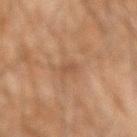Part of a total-body skin-imaging series; this lesion was reviewed on a skin check and was not flagged for biopsy. The lesion's longest dimension is about 2.5 mm. An algorithmic analysis of the crop reported an eccentricity of roughly 0.9 and a symmetry-axis asymmetry near 0.35. It also reported a lesion color around L≈41 a*≈17 b*≈27 in CIELAB and roughly 6 lightness units darker than nearby skin. A roughly 15 mm field-of-view crop from a total-body skin photograph. A male subject, roughly 45 years of age. From the left forearm.